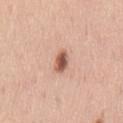<tbp_lesion>
  <biopsy_status>not biopsied; imaged during a skin examination</biopsy_status>
  <automated_metrics>
    <area_mm2_approx>4.0</area_mm2_approx>
    <cielab_L>56</cielab_L>
    <cielab_a>23</cielab_a>
    <cielab_b>29</cielab_b>
    <vs_skin_darker_L>16.0</vs_skin_darker_L>
    <vs_skin_contrast_norm>10.0</vs_skin_contrast_norm>
  </automated_metrics>
  <image>
    <source>total-body photography crop</source>
    <field_of_view_mm>15</field_of_view_mm>
  </image>
  <lighting>white-light</lighting>
  <patient>
    <sex>male</sex>
    <age_approx>40</age_approx>
  </patient>
  <lesion_size>
    <long_diameter_mm_approx>3.0</long_diameter_mm_approx>
  </lesion_size>
  <site>back</site>
</tbp_lesion>